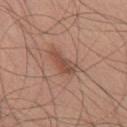Part of a total-body skin-imaging series; this lesion was reviewed on a skin check and was not flagged for biopsy. Longest diameter approximately 4 mm. A male patient aged 28 to 32. Cropped from a whole-body photographic skin survey; the tile spans about 15 mm. The lesion is on the chest.